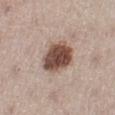biopsy status: total-body-photography surveillance lesion; no biopsy | patient: female, roughly 50 years of age | image-analysis metrics: an average lesion color of about L≈48 a*≈18 b*≈24 (CIELAB) and about 19 CIELAB-L* units darker than the surrounding skin; a lesion-detection confidence of about 100/100 | image source: ~15 mm tile from a whole-body skin photo | lighting: white-light | size: about 4.5 mm | anatomic site: the left lower leg.